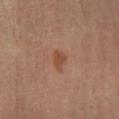Notes:
- imaging modality — total-body-photography crop, ~15 mm field of view
- body site — the right lower leg
- lesion diameter — ≈2.5 mm
- patient — aged around 55